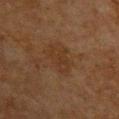– biopsy status: total-body-photography surveillance lesion; no biopsy
– body site: the upper back
– subject: male, about 65 years old
– illumination: cross-polarized
– TBP lesion metrics: an outline eccentricity of about 0.7 (0 = round, 1 = elongated) and a symmetry-axis asymmetry near 0.35; a lesion–skin lightness drop of about 4 and a normalized border contrast of about 5; a classifier nevus-likeness of about 0/100 and a detector confidence of about 100 out of 100 that the crop contains a lesion
– acquisition: ~15 mm tile from a whole-body skin photo
– size: ~3.5 mm (longest diameter)